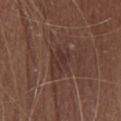| key | value |
|---|---|
| biopsy status | total-body-photography surveillance lesion; no biopsy |
| acquisition | ~15 mm tile from a whole-body skin photo |
| patient | female, aged 53 to 57 |
| body site | the head or neck |